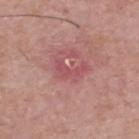Q: Was a biopsy performed?
A: no biopsy performed (imaged during a skin exam)
Q: How was this image acquired?
A: ~15 mm crop, total-body skin-cancer survey
Q: Who is the patient?
A: male, aged approximately 65
Q: Lesion location?
A: the back